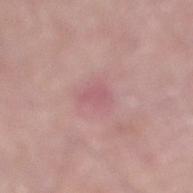Imaged during a routine full-body skin examination; the lesion was not biopsied and no histopathology is available. On the right lower leg. A male patient, aged 58–62. A roughly 15 mm field-of-view crop from a total-body skin photograph.